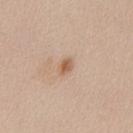The lesion was photographed on a routine skin check and not biopsied; there is no pathology result.
The lesion is on the front of the torso.
Cropped from a total-body skin-imaging series; the visible field is about 15 mm.
The subject is a female aged approximately 20.
Captured under white-light illumination.
The lesion's longest dimension is about 2 mm.
Automated image analysis of the tile measured an eccentricity of roughly 0.65 and a symmetry-axis asymmetry near 0.15. And it measured a mean CIELAB color near L≈59 a*≈19 b*≈33, a lesion–skin lightness drop of about 11, and a normalized border contrast of about 8. The software also gave border irregularity of about 1 on a 0–10 scale and radial color variation of about 0.5. And it measured an automated nevus-likeness rating near 75 out of 100.